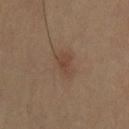Captured during whole-body skin photography for melanoma surveillance; the lesion was not biopsied.
This image is a 15 mm lesion crop taken from a total-body photograph.
The lesion is located on the upper back.
The patient is a male in their mid- to late 30s.
Longest diameter approximately 3 mm.
Captured under cross-polarized illumination.
Automated tile analysis of the lesion measured a border-irregularity rating of about 3/10, a within-lesion color-variation index near 2/10, and a peripheral color-asymmetry measure near 0.5.